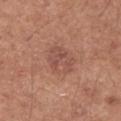biopsy_status: not biopsied; imaged during a skin examination
lighting: white-light
image:
  source: total-body photography crop
  field_of_view_mm: 15
patient:
  sex: male
  age_approx: 55
site: arm